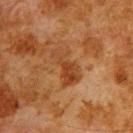Q: Was this lesion biopsied?
A: no biopsy performed (imaged during a skin exam)
Q: What is the imaging modality?
A: ~15 mm tile from a whole-body skin photo
Q: Where on the body is the lesion?
A: the upper back
Q: Patient demographics?
A: male, aged approximately 80